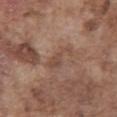Q: What is the anatomic site?
A: the abdomen
Q: Patient demographics?
A: male, about 75 years old
Q: How was the tile lit?
A: white-light illumination
Q: What kind of image is this?
A: ~15 mm tile from a whole-body skin photo
Q: What is the lesion's diameter?
A: ~4 mm (longest diameter)